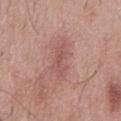biopsy_status: not biopsied; imaged during a skin examination
image:
  source: total-body photography crop
  field_of_view_mm: 15
automated_metrics:
  area_mm2_approx: 6.0
  eccentricity: 0.95
  shape_asymmetry: 0.3
  cielab_L: 54
  cielab_a: 23
  cielab_b: 24
  vs_skin_contrast_norm: 5.5
  border_irregularity_0_10: 4.0
  color_variation_0_10: 2.0
  peripheral_color_asymmetry: 0.5
patient:
  sex: male
  age_approx: 55
site: mid back
lesion_size:
  long_diameter_mm_approx: 4.5
lighting: white-light